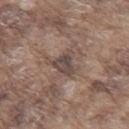Part of a total-body skin-imaging series; this lesion was reviewed on a skin check and was not flagged for biopsy. A male subject, about 75 years old. Approximately 3.5 mm at its widest. A 15 mm close-up tile from a total-body photography series done for melanoma screening. From the mid back.